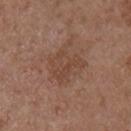{"biopsy_status": "not biopsied; imaged during a skin examination", "patient": {"sex": "male", "age_approx": 55}, "image": {"source": "total-body photography crop", "field_of_view_mm": 15}, "lighting": "white-light", "site": "right upper arm", "lesion_size": {"long_diameter_mm_approx": 4.5}}